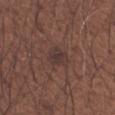Clinical impression:
The lesion was photographed on a routine skin check and not biopsied; there is no pathology result.
Context:
A close-up tile cropped from a whole-body skin photograph, about 15 mm across. Located on the left forearm. Automated image analysis of the tile measured a lesion–skin lightness drop of about 6. And it measured a border-irregularity index near 3/10 and a color-variation rating of about 2/10. And it measured a classifier nevus-likeness of about 0/100 and a lesion-detection confidence of about 100/100. About 3 mm across. A male patient about 65 years old.